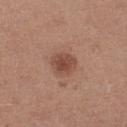The lesion was photographed on a routine skin check and not biopsied; there is no pathology result. A female patient in their mid-40s. The tile uses white-light illumination. A region of skin cropped from a whole-body photographic capture, roughly 15 mm wide. Longest diameter approximately 3 mm. On the left thigh.